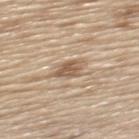Captured during whole-body skin photography for melanoma surveillance; the lesion was not biopsied. The lesion's longest dimension is about 3.5 mm. From the upper back. A male subject, aged approximately 70. A 15 mm close-up tile from a total-body photography series done for melanoma screening. Automated tile analysis of the lesion measured an average lesion color of about L≈57 a*≈15 b*≈30 (CIELAB) and a lesion-to-skin contrast of about 7.5 (normalized; higher = more distinct). And it measured a border-irregularity rating of about 3/10, internal color variation of about 3 on a 0–10 scale, and peripheral color asymmetry of about 1. And it measured a lesion-detection confidence of about 65/100. The tile uses white-light illumination.